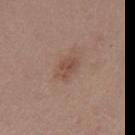{"biopsy_status": "not biopsied; imaged during a skin examination", "image": {"source": "total-body photography crop", "field_of_view_mm": 15}, "site": "mid back", "patient": {"sex": "female", "age_approx": 50}}